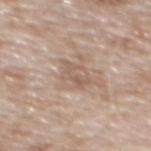biopsy status: imaged on a skin check; not biopsied
site: the mid back
patient: male, in their 80s
imaging modality: ~15 mm tile from a whole-body skin photo
illumination: white-light illumination
diameter: ≈3 mm
TBP lesion metrics: a footprint of about 5.5 mm², an outline eccentricity of about 0.65 (0 = round, 1 = elongated), and two-axis asymmetry of about 0.3; border irregularity of about 3.5 on a 0–10 scale and a color-variation rating of about 4/10